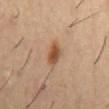Assessment: No biopsy was performed on this lesion — it was imaged during a full skin examination and was not determined to be concerning. Acquisition and patient details: The subject is a male approximately 55 years of age. Captured under cross-polarized illumination. The recorded lesion diameter is about 3 mm. Automated image analysis of the tile measured an automated nevus-likeness rating near 95 out of 100 and lesion-presence confidence of about 100/100. A roughly 15 mm field-of-view crop from a total-body skin photograph. From the front of the torso.